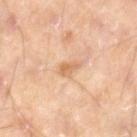Impression: The lesion was photographed on a routine skin check and not biopsied; there is no pathology result. Context: The recorded lesion diameter is about 2.5 mm. An algorithmic analysis of the crop reported an area of roughly 3.5 mm², an eccentricity of roughly 0.8, and two-axis asymmetry of about 0.3. The software also gave an average lesion color of about L≈66 a*≈20 b*≈37 (CIELAB), roughly 9 lightness units darker than nearby skin, and a normalized lesion–skin contrast near 6.5. It also reported a lesion-detection confidence of about 100/100. On the right lower leg. A male patient approximately 45 years of age. This image is a 15 mm lesion crop taken from a total-body photograph.